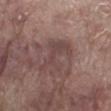Part of a total-body skin-imaging series; this lesion was reviewed on a skin check and was not flagged for biopsy. Located on the right lower leg. A male subject, aged around 70. This is a white-light tile. Cropped from a whole-body photographic skin survey; the tile spans about 15 mm.